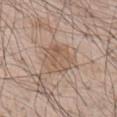Impression:
Recorded during total-body skin imaging; not selected for excision or biopsy.